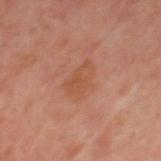Imaged during a routine full-body skin examination; the lesion was not biopsied and no histopathology is available. The patient is a male aged 63 to 67. Approximately 4 mm at its widest. A roughly 15 mm field-of-view crop from a total-body skin photograph. Located on the mid back. This is a cross-polarized tile.